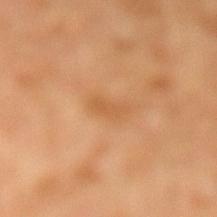Notes:
* lesion diameter: about 3 mm
* image source: 15 mm crop, total-body photography
* patient: male, aged 28 to 32
* illumination: cross-polarized
* location: the left lower leg